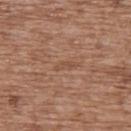The lesion was tiled from a total-body skin photograph and was not biopsied. A roughly 15 mm field-of-view crop from a total-body skin photograph. From the upper back. A female subject, aged around 75. The tile uses white-light illumination.